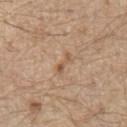Case summary:
– biopsy status · catalogued during a skin exam; not biopsied
– lesion size · ≈3 mm
– image · total-body-photography crop, ~15 mm field of view
– patient · male, aged 68 to 72
– location · the chest